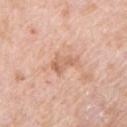{
  "biopsy_status": "not biopsied; imaged during a skin examination",
  "automated_metrics": {
    "area_mm2_approx": 5.0,
    "eccentricity": 0.85,
    "cielab_L": 64,
    "cielab_a": 22,
    "cielab_b": 32,
    "vs_skin_darker_L": 9.0,
    "vs_skin_contrast_norm": 5.5
  },
  "site": "left upper arm",
  "image": {
    "source": "total-body photography crop",
    "field_of_view_mm": 15
  },
  "patient": {
    "sex": "male",
    "age_approx": 50
  }
}